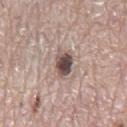Findings:
• notes: imaged on a skin check; not biopsied
• subject: male, aged 73–77
• lighting: white-light
• location: the mid back
• image source: ~15 mm crop, total-body skin-cancer survey
• lesion diameter: ≈4 mm